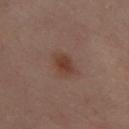{"biopsy_status": "not biopsied; imaged during a skin examination", "site": "right leg", "image": {"source": "total-body photography crop", "field_of_view_mm": 15}, "patient": {"sex": "female", "age_approx": 60}, "automated_metrics": {"vs_skin_contrast_norm": 7.5, "nevus_likeness_0_100": 95}}